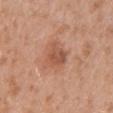biopsy status = total-body-photography surveillance lesion; no biopsy
body site = the left upper arm
subject = female, about 30 years old
image = ~15 mm tile from a whole-body skin photo
diameter = about 3 mm
automated lesion analysis = a lesion area of about 6 mm², a shape eccentricity near 0.6, and a symmetry-axis asymmetry near 0.3; an automated nevus-likeness rating near 20 out of 100 and a lesion-detection confidence of about 100/100